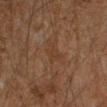Notes:
- biopsy status — catalogued during a skin exam; not biopsied
- TBP lesion metrics — a mean CIELAB color near L≈29 a*≈15 b*≈25, a lesion–skin lightness drop of about 4, and a lesion-to-skin contrast of about 4.5 (normalized; higher = more distinct)
- body site — the left forearm
- lesion diameter — ≈2.5 mm
- acquisition — ~15 mm tile from a whole-body skin photo
- patient — male, in their mid- to late 40s
- illumination — cross-polarized illumination